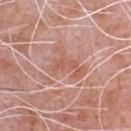The lesion was tiled from a total-body skin photograph and was not biopsied. The total-body-photography lesion software estimated a lesion area of about 11 mm² and a shape eccentricity near 0.75. The subject is a male aged approximately 80. A lesion tile, about 15 mm wide, cut from a 3D total-body photograph. The lesion is on the chest. About 5 mm across. The tile uses white-light illumination.